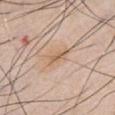Q: Is there a histopathology result?
A: no biopsy performed (imaged during a skin exam)
Q: How large is the lesion?
A: about 3 mm
Q: Automated lesion metrics?
A: a detector confidence of about 75 out of 100 that the crop contains a lesion
Q: What are the patient's age and sex?
A: male, aged 43 to 47
Q: What is the anatomic site?
A: the chest
Q: How was this image acquired?
A: ~15 mm tile from a whole-body skin photo